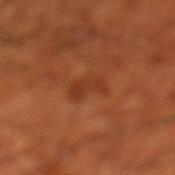Case summary:
– biopsy status: imaged on a skin check; not biopsied
– patient: male, about 70 years old
– site: the left lower leg
– lighting: cross-polarized illumination
– imaging modality: total-body-photography crop, ~15 mm field of view
– size: ~3 mm (longest diameter)